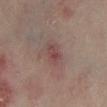notes=no biopsy performed (imaged during a skin exam) | subject=female, aged 33–37 | acquisition=~15 mm crop, total-body skin-cancer survey | location=the left lower leg | diameter=≈3 mm.